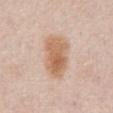Assessment:
Recorded during total-body skin imaging; not selected for excision or biopsy.
Clinical summary:
The lesion is located on the abdomen. The tile uses white-light illumination. Automated tile analysis of the lesion measured a lesion color around L≈63 a*≈19 b*≈32 in CIELAB, roughly 11 lightness units darker than nearby skin, and a normalized lesion–skin contrast near 8. The analysis additionally found border irregularity of about 2 on a 0–10 scale, internal color variation of about 4.5 on a 0–10 scale, and radial color variation of about 1.5. The analysis additionally found a classifier nevus-likeness of about 95/100. Approximately 5.5 mm at its widest. A lesion tile, about 15 mm wide, cut from a 3D total-body photograph. A male subject, aged around 75.